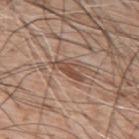Clinical summary:
The tile uses white-light illumination. A roughly 15 mm field-of-view crop from a total-body skin photograph. A male subject roughly 60 years of age. The lesion is located on the upper back.